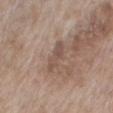Impression:
This lesion was catalogued during total-body skin photography and was not selected for biopsy.
Background:
The recorded lesion diameter is about 4 mm. This image is a 15 mm lesion crop taken from a total-body photograph. Automated image analysis of the tile measured a lesion area of about 5 mm². And it measured a border-irregularity rating of about 4/10. The software also gave a classifier nevus-likeness of about 0/100 and a detector confidence of about 70 out of 100 that the crop contains a lesion. The lesion is located on the back. The patient is a female in their mid- to late 70s.